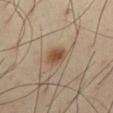notes: catalogued during a skin exam; not biopsied